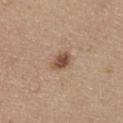{
  "biopsy_status": "not biopsied; imaged during a skin examination",
  "site": "chest",
  "lighting": "white-light",
  "lesion_size": {
    "long_diameter_mm_approx": 2.5
  },
  "image": {
    "source": "total-body photography crop",
    "field_of_view_mm": 15
  },
  "patient": {
    "sex": "female",
    "age_approx": 65
  }
}